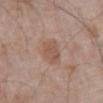  biopsy_status: not biopsied; imaged during a skin examination
  patient:
    sex: male
    age_approx: 70
  automated_metrics:
    nevus_likeness_0_100: 40
  lesion_size:
    long_diameter_mm_approx: 3.5
  lighting: white-light
  image:
    source: total-body photography crop
    field_of_view_mm: 15
  site: abdomen